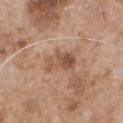Q: Was a biopsy performed?
A: imaged on a skin check; not biopsied
Q: Illumination type?
A: white-light illumination
Q: What are the patient's age and sex?
A: male, about 65 years old
Q: What is the anatomic site?
A: the chest
Q: What kind of image is this?
A: ~15 mm crop, total-body skin-cancer survey
Q: What is the lesion's diameter?
A: about 4 mm